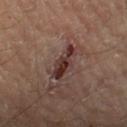Imaged during a routine full-body skin examination; the lesion was not biopsied and no histopathology is available. Approximately 4 mm at its widest. From the left thigh. Captured under cross-polarized illumination. Automated image analysis of the tile measured a lesion area of about 5.5 mm² and two-axis asymmetry of about 0.35. And it measured a lesion color around L≈32 a*≈21 b*≈20 in CIELAB and a normalized border contrast of about 11.5. Cropped from a whole-body photographic skin survey; the tile spans about 15 mm. A male patient, approximately 65 years of age.